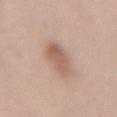image source: ~15 mm tile from a whole-body skin photo; anatomic site: the abdomen; patient: female, aged around 65.